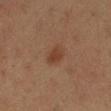Q: Is there a histopathology result?
A: imaged on a skin check; not biopsied
Q: Who is the patient?
A: female, about 40 years old
Q: What is the lesion's diameter?
A: ≈2.5 mm
Q: What lighting was used for the tile?
A: cross-polarized illumination
Q: Where on the body is the lesion?
A: the left lower leg
Q: What kind of image is this?
A: ~15 mm tile from a whole-body skin photo